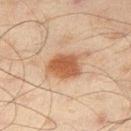Q: Was this lesion biopsied?
A: total-body-photography surveillance lesion; no biopsy
Q: Who is the patient?
A: male, aged 43–47
Q: How was this image acquired?
A: ~15 mm crop, total-body skin-cancer survey
Q: How large is the lesion?
A: ≈4 mm
Q: Where on the body is the lesion?
A: the right thigh